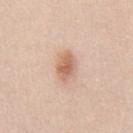Captured during whole-body skin photography for melanoma surveillance; the lesion was not biopsied.
Measured at roughly 4 mm in maximum diameter.
Cropped from a whole-body photographic skin survey; the tile spans about 15 mm.
A male subject, about 35 years old.
An algorithmic analysis of the crop reported a shape eccentricity near 0.8. The software also gave a border-irregularity rating of about 2/10 and a within-lesion color-variation index near 4.5/10.
From the abdomen.